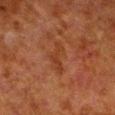Impression:
Captured during whole-body skin photography for melanoma surveillance; the lesion was not biopsied.
Background:
A male patient aged around 80. Located on the left lower leg. Cropped from a total-body skin-imaging series; the visible field is about 15 mm.